On the leg; a region of skin cropped from a whole-body photographic capture, roughly 15 mm wide; a male patient roughly 60 years of age:
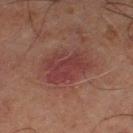On excision, pathology confirmed a superficial basal cell carcinoma.